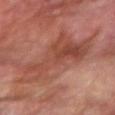notes: catalogued during a skin exam; not biopsied | size: ~8.5 mm (longest diameter) | lighting: cross-polarized | subject: male, about 70 years old | automated metrics: an eccentricity of roughly 0.85 and two-axis asymmetry of about 0.55; border irregularity of about 8.5 on a 0–10 scale and a color-variation rating of about 5/10 | location: the arm | image: total-body-photography crop, ~15 mm field of view.